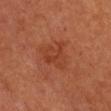biopsy status = imaged on a skin check; not biopsied | anatomic site = the chest | subject = female, aged 63–67 | diameter = ≈3.5 mm | tile lighting = cross-polarized | image source = ~15 mm crop, total-body skin-cancer survey.